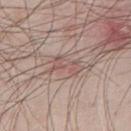follow-up: no biopsy performed (imaged during a skin exam) | diameter: about 4 mm | site: the left upper arm | image: total-body-photography crop, ~15 mm field of view | illumination: white-light | subject: male, in their mid-40s.